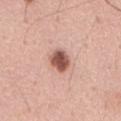The lesion was photographed on a routine skin check and not biopsied; there is no pathology result. Longest diameter approximately 2.5 mm. The lesion-visualizer software estimated a footprint of about 6 mm², a shape eccentricity near 0.5, and a shape-asymmetry score of about 0.15 (0 = symmetric). And it measured a lesion color around L≈54 a*≈24 b*≈26 in CIELAB and a normalized border contrast of about 11. The software also gave an automated nevus-likeness rating near 95 out of 100. Located on the front of the torso. The subject is a male aged approximately 60. A 15 mm close-up tile from a total-body photography series done for melanoma screening.